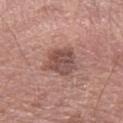Recorded during total-body skin imaging; not selected for excision or biopsy. A male patient approximately 55 years of age. This is a white-light tile. Approximately 4 mm at its widest. A roughly 15 mm field-of-view crop from a total-body skin photograph. The lesion-visualizer software estimated an average lesion color of about L≈50 a*≈21 b*≈23 (CIELAB), about 11 CIELAB-L* units darker than the surrounding skin, and a normalized border contrast of about 8. It also reported a border-irregularity rating of about 3/10, internal color variation of about 4 on a 0–10 scale, and peripheral color asymmetry of about 1.5. It also reported a nevus-likeness score of about 5/100 and a lesion-detection confidence of about 100/100. On the left forearm.